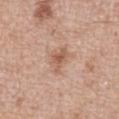No biopsy was performed on this lesion — it was imaged during a full skin examination and was not determined to be concerning. Measured at roughly 3 mm in maximum diameter. Located on the abdomen. A 15 mm close-up extracted from a 3D total-body photography capture. Imaged with white-light lighting. A male subject about 50 years old.